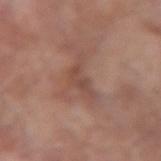| field | value |
|---|---|
| biopsy status | total-body-photography surveillance lesion; no biopsy |
| site | the left forearm |
| image | ~15 mm crop, total-body skin-cancer survey |
| lesion size | about 3 mm |
| subject | male, aged approximately 55 |
| lighting | white-light illumination |